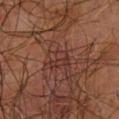Q: Is there a histopathology result?
A: no biopsy performed (imaged during a skin exam)
Q: What kind of image is this?
A: total-body-photography crop, ~15 mm field of view
Q: Illumination type?
A: cross-polarized illumination
Q: Lesion location?
A: the right upper arm
Q: Lesion size?
A: ~3 mm (longest diameter)
Q: Patient demographics?
A: male, aged 63–67